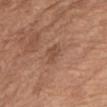follow-up = catalogued during a skin exam; not biopsied | acquisition = ~15 mm tile from a whole-body skin photo | location = the abdomen | size = ~3 mm (longest diameter) | subject = female, about 65 years old.